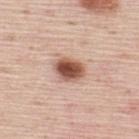Assessment:
Captured during whole-body skin photography for melanoma surveillance; the lesion was not biopsied.
Clinical summary:
This is a white-light tile. Approximately 3.5 mm at its widest. From the upper back. Automated tile analysis of the lesion measured a mean CIELAB color near L≈54 a*≈22 b*≈28 and about 19 CIELAB-L* units darker than the surrounding skin. It also reported a classifier nevus-likeness of about 100/100 and a detector confidence of about 100 out of 100 that the crop contains a lesion. Cropped from a whole-body photographic skin survey; the tile spans about 15 mm. The subject is a male approximately 45 years of age.